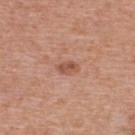Recorded during total-body skin imaging; not selected for excision or biopsy. A 15 mm crop from a total-body photograph taken for skin-cancer surveillance. The tile uses white-light illumination. The recorded lesion diameter is about 2.5 mm. Located on the back. The patient is a female roughly 40 years of age.